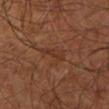The lesion was photographed on a routine skin check and not biopsied; there is no pathology result.
Longest diameter approximately 3 mm.
Captured under cross-polarized illumination.
Located on the left lower leg.
A subject approximately 65 years of age.
Automated tile analysis of the lesion measured a lesion area of about 4.5 mm², an outline eccentricity of about 0.8 (0 = round, 1 = elongated), and a symmetry-axis asymmetry near 0.45. It also reported a lesion color around L≈34 a*≈21 b*≈28 in CIELAB and a normalized border contrast of about 5. The analysis additionally found border irregularity of about 5 on a 0–10 scale, a color-variation rating of about 2/10, and peripheral color asymmetry of about 1. And it measured a classifier nevus-likeness of about 0/100 and a lesion-detection confidence of about 95/100.
A region of skin cropped from a whole-body photographic capture, roughly 15 mm wide.